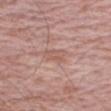Impression:
This lesion was catalogued during total-body skin photography and was not selected for biopsy.
Background:
From the right forearm. The tile uses white-light illumination. The lesion's longest dimension is about 3 mm. An algorithmic analysis of the crop reported two-axis asymmetry of about 0.3. It also reported a mean CIELAB color near L≈57 a*≈21 b*≈25, about 7 CIELAB-L* units darker than the surrounding skin, and a normalized border contrast of about 5. Cropped from a whole-body photographic skin survey; the tile spans about 15 mm. A male subject, in their mid-70s.